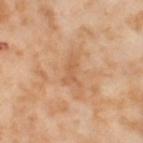{"biopsy_status": "not biopsied; imaged during a skin examination", "lighting": "cross-polarized", "image": {"source": "total-body photography crop", "field_of_view_mm": 15}, "site": "left thigh", "patient": {"sex": "female", "age_approx": 55}, "lesion_size": {"long_diameter_mm_approx": 3.5}}